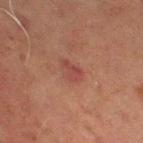Clinical impression:
Captured during whole-body skin photography for melanoma surveillance; the lesion was not biopsied.
Image and clinical context:
A male patient, in their 70s. A region of skin cropped from a whole-body photographic capture, roughly 15 mm wide. Automated tile analysis of the lesion measured a footprint of about 5 mm² and an outline eccentricity of about 0.8 (0 = round, 1 = elongated). The analysis additionally found an average lesion color of about L≈37 a*≈23 b*≈22 (CIELAB), a lesion–skin lightness drop of about 6, and a normalized lesion–skin contrast near 5.5. The analysis additionally found a within-lesion color-variation index near 3/10 and a peripheral color-asymmetry measure near 1. Imaged with cross-polarized lighting. The lesion is on the left thigh.